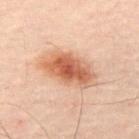{"biopsy_status": "not biopsied; imaged during a skin examination", "lighting": "cross-polarized", "image": {"source": "total-body photography crop", "field_of_view_mm": 15}, "site": "left thigh", "patient": {"sex": "male", "age_approx": 50}}